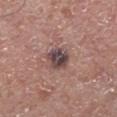workup — no biopsy performed (imaged during a skin exam) | image source — ~15 mm crop, total-body skin-cancer survey | subject — male, aged around 55 | body site — the right lower leg.